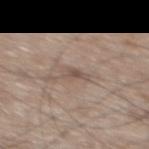Q: What lighting was used for the tile?
A: white-light illumination
Q: What kind of image is this?
A: total-body-photography crop, ~15 mm field of view
Q: What is the lesion's diameter?
A: about 2.5 mm
Q: Who is the patient?
A: male, roughly 75 years of age
Q: Where on the body is the lesion?
A: the back